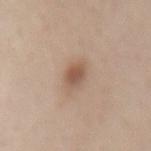{
  "biopsy_status": "not biopsied; imaged during a skin examination",
  "patient": {
    "sex": "male",
    "age_approx": 40
  },
  "image": {
    "source": "total-body photography crop",
    "field_of_view_mm": 15
  },
  "site": "lower back"
}